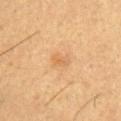| key | value |
|---|---|
| follow-up | catalogued during a skin exam; not biopsied |
| image | total-body-photography crop, ~15 mm field of view |
| location | the upper back |
| patient | male, roughly 55 years of age |
| tile lighting | cross-polarized |
| automated metrics | a lesion area of about 4 mm² and an eccentricity of roughly 0.75; a lesion-to-skin contrast of about 4.5 (normalized; higher = more distinct); a border-irregularity index near 3/10, a color-variation rating of about 2/10, and a peripheral color-asymmetry measure near 0.5; a classifier nevus-likeness of about 0/100 and a detector confidence of about 100 out of 100 that the crop contains a lesion |